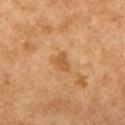Clinical impression:
Imaged during a routine full-body skin examination; the lesion was not biopsied and no histopathology is available.
Context:
This is a cross-polarized tile. A 15 mm close-up extracted from a 3D total-body photography capture. Automated image analysis of the tile measured a symmetry-axis asymmetry near 0.35. And it measured a border-irregularity index near 3/10 and a peripheral color-asymmetry measure near 0.5. The software also gave an automated nevus-likeness rating near 10 out of 100 and lesion-presence confidence of about 100/100. The lesion's longest dimension is about 2.5 mm. On the left upper arm. A female patient aged approximately 60.